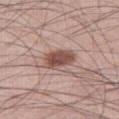Assessment: The lesion was photographed on a routine skin check and not biopsied; there is no pathology result. Clinical summary: Imaged with white-light lighting. On the right thigh. About 4 mm across. The subject is a male aged approximately 45. Cropped from a whole-body photographic skin survey; the tile spans about 15 mm. Automated tile analysis of the lesion measured an average lesion color of about L≈50 a*≈20 b*≈24 (CIELAB) and a lesion-to-skin contrast of about 10 (normalized; higher = more distinct). It also reported a border-irregularity index near 2/10, internal color variation of about 3.5 on a 0–10 scale, and radial color variation of about 1.